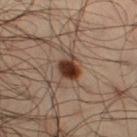Assessment:
The lesion was photographed on a routine skin check and not biopsied; there is no pathology result.
Background:
Measured at roughly 3.5 mm in maximum diameter. From the right thigh. Cropped from a whole-body photographic skin survey; the tile spans about 15 mm. The patient is a male aged 33 to 37. This is a cross-polarized tile.